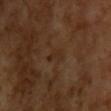Impression:
Part of a total-body skin-imaging series; this lesion was reviewed on a skin check and was not flagged for biopsy.